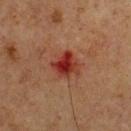| feature | finding |
|---|---|
| workup | no biopsy performed (imaged during a skin exam) |
| subject | male, about 75 years old |
| image source | total-body-photography crop, ~15 mm field of view |
| anatomic site | the chest |
| size | ~3 mm (longest diameter) |
| automated metrics | a lesion area of about 7.5 mm² and a shape-asymmetry score of about 0.25 (0 = symmetric); a nevus-likeness score of about 0/100 |
| illumination | cross-polarized illumination |